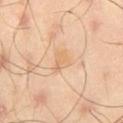notes = total-body-photography surveillance lesion; no biopsy | illumination = cross-polarized | patient = male, aged approximately 45 | site = the right thigh | imaging modality = 15 mm crop, total-body photography | size = about 2.5 mm.